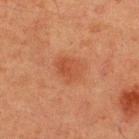{"biopsy_status": "not biopsied; imaged during a skin examination", "automated_metrics": {"cielab_L": 39, "cielab_a": 23, "cielab_b": 31, "vs_skin_darker_L": 6.0, "vs_skin_contrast_norm": 5.5, "nevus_likeness_0_100": 50, "lesion_detection_confidence_0_100": 100}, "image": {"source": "total-body photography crop", "field_of_view_mm": 15}, "lesion_size": {"long_diameter_mm_approx": 3.0}, "lighting": "cross-polarized", "site": "upper back", "patient": {"sex": "male", "age_approx": 60}}